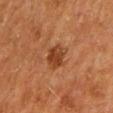Q: Where on the body is the lesion?
A: the mid back
Q: What are the patient's age and sex?
A: male, aged 63–67
Q: How was the tile lit?
A: cross-polarized
Q: What is the imaging modality?
A: total-body-photography crop, ~15 mm field of view
Q: Lesion size?
A: ≈3.5 mm